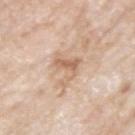The lesion was tiled from a total-body skin photograph and was not biopsied. Located on the right upper arm. The subject is a male about 75 years old. This image is a 15 mm lesion crop taken from a total-body photograph.